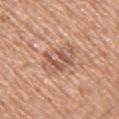{"biopsy_status": "not biopsied; imaged during a skin examination", "patient": {"sex": "male", "age_approx": 65}, "image": {"source": "total-body photography crop", "field_of_view_mm": 15}, "lesion_size": {"long_diameter_mm_approx": 4.0}, "site": "right upper arm"}